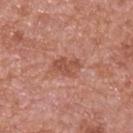Imaged during a routine full-body skin examination; the lesion was not biopsied and no histopathology is available. On the upper back. The patient is a male roughly 65 years of age. Captured under white-light illumination. Measured at roughly 3 mm in maximum diameter. Cropped from a total-body skin-imaging series; the visible field is about 15 mm.